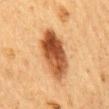Part of a total-body skin-imaging series; this lesion was reviewed on a skin check and was not flagged for biopsy. This is a cross-polarized tile. The lesion's longest dimension is about 6.5 mm. The lesion is on the mid back. A male subject aged 58 to 62. This image is a 15 mm lesion crop taken from a total-body photograph.